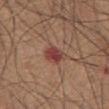follow-up: catalogued during a skin exam; not biopsied
diameter: ~3.5 mm (longest diameter)
TBP lesion metrics: a footprint of about 6 mm² and an outline eccentricity of about 0.75 (0 = round, 1 = elongated); a lesion color around L≈43 a*≈25 b*≈25 in CIELAB, about 10 CIELAB-L* units darker than the surrounding skin, and a normalized border contrast of about 8.5
image: total-body-photography crop, ~15 mm field of view
illumination: white-light illumination
subject: male, in their mid-70s
body site: the abdomen